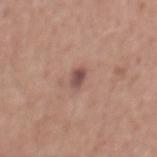{"biopsy_status": "not biopsied; imaged during a skin examination", "lesion_size": {"long_diameter_mm_approx": 2.5}, "patient": {"sex": "male", "age_approx": 55}, "automated_metrics": {"eccentricity": 0.8, "shape_asymmetry": 0.2, "border_irregularity_0_10": 2.0, "peripheral_color_asymmetry": 1.5}, "image": {"source": "total-body photography crop", "field_of_view_mm": 15}, "site": "mid back", "lighting": "white-light"}